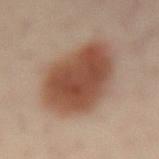biopsy status: catalogued during a skin exam; not biopsied
TBP lesion metrics: a shape eccentricity near 0.65 and a shape-asymmetry score of about 0.15 (0 = symmetric); a mean CIELAB color near L≈47 a*≈20 b*≈28, roughly 14 lightness units darker than nearby skin, and a lesion-to-skin contrast of about 10.5 (normalized; higher = more distinct); a border-irregularity index near 1.5/10, a within-lesion color-variation index near 4.5/10, and radial color variation of about 1.5; a classifier nevus-likeness of about 100/100 and lesion-presence confidence of about 100/100
lesion diameter: ≈8 mm
illumination: cross-polarized illumination
location: the lower back
image: total-body-photography crop, ~15 mm field of view
patient: male, aged 38 to 42